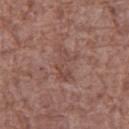Q: Was this lesion biopsied?
A: total-body-photography surveillance lesion; no biopsy
Q: How was the tile lit?
A: white-light illumination
Q: Who is the patient?
A: female, aged 73–77
Q: What kind of image is this?
A: 15 mm crop, total-body photography
Q: What is the anatomic site?
A: the right thigh
Q: Lesion size?
A: ≈4.5 mm